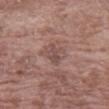- workup · total-body-photography surveillance lesion; no biopsy
- image · ~15 mm crop, total-body skin-cancer survey
- subject · female, about 70 years old
- body site · the left forearm